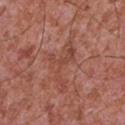{
  "biopsy_status": "not biopsied; imaged during a skin examination",
  "site": "chest",
  "lesion_size": {
    "long_diameter_mm_approx": 8.0
  },
  "automated_metrics": {
    "area_mm2_approx": 13.0,
    "eccentricity": 0.9,
    "shape_asymmetry": 0.7,
    "cielab_L": 47,
    "cielab_a": 25,
    "cielab_b": 28,
    "vs_skin_darker_L": 6.0,
    "vs_skin_contrast_norm": 4.5,
    "border_irregularity_0_10": 10.0,
    "color_variation_0_10": 3.0,
    "peripheral_color_asymmetry": 1.0,
    "nevus_likeness_0_100": 0
  },
  "image": {
    "source": "total-body photography crop",
    "field_of_view_mm": 15
  },
  "patient": {
    "sex": "male",
    "age_approx": 45
  },
  "lighting": "white-light"
}